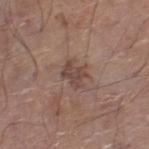Notes:
- biopsy status: no biopsy performed (imaged during a skin exam)
- image: total-body-photography crop, ~15 mm field of view
- patient: male, aged 68 to 72
- size: about 3 mm
- anatomic site: the right lower leg
- illumination: white-light illumination